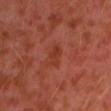notes=catalogued during a skin exam; not biopsied
image-analysis metrics=a border-irregularity index near 3/10, a color-variation rating of about 1.5/10, and radial color variation of about 0.5; a nevus-likeness score of about 0/100 and lesion-presence confidence of about 100/100
subject=male, in their 30s
image=~15 mm tile from a whole-body skin photo
site=the arm